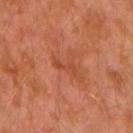Clinical impression: This lesion was catalogued during total-body skin photography and was not selected for biopsy. Background: A male patient, roughly 30 years of age. This is a cross-polarized tile. From the arm. A 15 mm crop from a total-body photograph taken for skin-cancer surveillance. About 2.5 mm across.